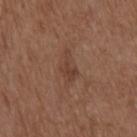workup: total-body-photography surveillance lesion; no biopsy | tile lighting: white-light | size: ≈3.5 mm | acquisition: ~15 mm tile from a whole-body skin photo | patient: male, aged 73 to 77 | body site: the chest | automated lesion analysis: a mean CIELAB color near L≈40 a*≈19 b*≈27, roughly 7 lightness units darker than nearby skin, and a normalized border contrast of about 6; a color-variation rating of about 2/10; a classifier nevus-likeness of about 0/100 and a detector confidence of about 100 out of 100 that the crop contains a lesion.